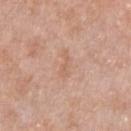Captured during whole-body skin photography for melanoma surveillance; the lesion was not biopsied.
The tile uses white-light illumination.
A male patient, aged 48–52.
About 3.5 mm across.
The total-body-photography lesion software estimated about 6 CIELAB-L* units darker than the surrounding skin and a normalized border contrast of about 4.5. The software also gave an automated nevus-likeness rating near 0 out of 100 and lesion-presence confidence of about 100/100.
A 15 mm crop from a total-body photograph taken for skin-cancer surveillance.
The lesion is located on the chest.